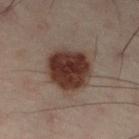Imaged during a routine full-body skin examination; the lesion was not biopsied and no histopathology is available. Located on the left lower leg. Measured at roughly 6 mm in maximum diameter. A male subject aged approximately 50. A 15 mm crop from a total-body photograph taken for skin-cancer surveillance. Automated image analysis of the tile measured a footprint of about 19 mm², an outline eccentricity of about 0.65 (0 = round, 1 = elongated), and a shape-asymmetry score of about 0.15 (0 = symmetric). And it measured a lesion color around L≈27 a*≈15 b*≈19 in CIELAB, a lesion–skin lightness drop of about 13, and a lesion-to-skin contrast of about 13.5 (normalized; higher = more distinct). Imaged with cross-polarized lighting.